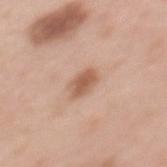Captured during whole-body skin photography for melanoma surveillance; the lesion was not biopsied. On the mid back. The recorded lesion diameter is about 3 mm. A female subject aged around 50. A 15 mm crop from a total-body photograph taken for skin-cancer surveillance. This is a white-light tile.